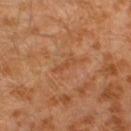biopsy status=total-body-photography surveillance lesion; no biopsy
body site=the left lower leg
illumination=cross-polarized
lesion size=≈3.5 mm
subject=male, aged approximately 30
imaging modality=total-body-photography crop, ~15 mm field of view
image-analysis metrics=an average lesion color of about L≈48 a*≈24 b*≈36 (CIELAB), a lesion–skin lightness drop of about 6, and a normalized border contrast of about 5; radial color variation of about 0; a classifier nevus-likeness of about 0/100 and lesion-presence confidence of about 95/100